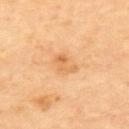Impression: The lesion was tiled from a total-body skin photograph and was not biopsied. Image and clinical context: The lesion is on the back. Captured under cross-polarized illumination. Approximately 3 mm at its widest. A 15 mm close-up tile from a total-body photography series done for melanoma screening.